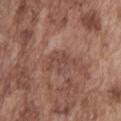<record>
  <biopsy_status>not biopsied; imaged during a skin examination</biopsy_status>
  <automated_metrics>
    <area_mm2_approx>4.0</area_mm2_approx>
    <shape_asymmetry>0.55</shape_asymmetry>
    <cielab_L>45</cielab_L>
    <cielab_a>21</cielab_a>
    <cielab_b>24</cielab_b>
  </automated_metrics>
  <image>
    <source>total-body photography crop</source>
    <field_of_view_mm>15</field_of_view_mm>
  </image>
  <site>front of the torso</site>
  <lesion_size>
    <long_diameter_mm_approx>2.5</long_diameter_mm_approx>
  </lesion_size>
  <patient>
    <sex>male</sex>
    <age_approx>75</age_approx>
  </patient>
</record>